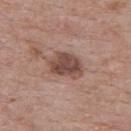  biopsy_status: not biopsied; imaged during a skin examination
  lighting: white-light
  automated_metrics:
    area_mm2_approx: 11.0
    eccentricity: 0.7
    shape_asymmetry: 0.2
    cielab_L: 47
    cielab_a: 19
    cielab_b: 24
    vs_skin_darker_L: 13.0
    vs_skin_contrast_norm: 9.0
    border_irregularity_0_10: 2.5
    color_variation_0_10: 4.0
    nevus_likeness_0_100: 70
    lesion_detection_confidence_0_100: 100
  site: upper back
  image:
    source: total-body photography crop
    field_of_view_mm: 15
  lesion_size:
    long_diameter_mm_approx: 4.5
  patient:
    sex: male
    age_approx: 70